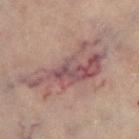Findings:
* biopsy status · no biopsy performed (imaged during a skin exam)
* patient · female, in their 60s
* diameter · about 10.5 mm
* location · the left thigh
* tile lighting · cross-polarized
* image-analysis metrics · an average lesion color of about L≈52 a*≈20 b*≈20 (CIELAB), a lesion–skin lightness drop of about 10, and a lesion-to-skin contrast of about 7.5 (normalized; higher = more distinct); a border-irregularity index near 8/10, internal color variation of about 8 on a 0–10 scale, and radial color variation of about 2.5; a classifier nevus-likeness of about 0/100 and a lesion-detection confidence of about 55/100
* image · ~15 mm tile from a whole-body skin photo